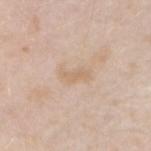The lesion was photographed on a routine skin check and not biopsied; there is no pathology result. This is a white-light tile. The lesion is located on the arm. A male subject, approximately 45 years of age. Automated tile analysis of the lesion measured an area of roughly 3.5 mm², an outline eccentricity of about 0.9 (0 = round, 1 = elongated), and a symmetry-axis asymmetry near 0.35. The software also gave a mean CIELAB color near L≈66 a*≈16 b*≈32, a lesion–skin lightness drop of about 6, and a normalized lesion–skin contrast near 5. The analysis additionally found an automated nevus-likeness rating near 0 out of 100 and lesion-presence confidence of about 100/100. A lesion tile, about 15 mm wide, cut from a 3D total-body photograph.